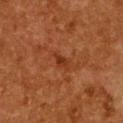| field | value |
|---|---|
| workup | imaged on a skin check; not biopsied |
| location | the chest |
| lighting | cross-polarized |
| image | ~15 mm tile from a whole-body skin photo |
| subject | female, aged 48 to 52 |
| lesion size | about 2.5 mm |